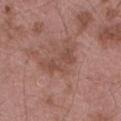Q: What lighting was used for the tile?
A: white-light illumination
Q: Lesion size?
A: ≈5 mm
Q: How was this image acquired?
A: ~15 mm tile from a whole-body skin photo
Q: Who is the patient?
A: male, aged approximately 55
Q: What is the anatomic site?
A: the lower back
Q: What did automated image analysis measure?
A: a lesion area of about 8.5 mm², an eccentricity of roughly 0.9, and two-axis asymmetry of about 0.5; a classifier nevus-likeness of about 0/100